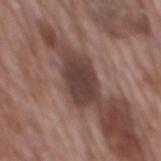notes: catalogued during a skin exam; not biopsied
body site: the mid back
image source: ~15 mm crop, total-body skin-cancer survey
subject: male, about 70 years old